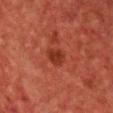workup: total-body-photography surveillance lesion; no biopsy
automated lesion analysis: a lesion area of about 4 mm², an eccentricity of roughly 0.6, and a shape-asymmetry score of about 0.2 (0 = symmetric); a nevus-likeness score of about 5/100 and a detector confidence of about 100 out of 100 that the crop contains a lesion
subject: male, approximately 50 years of age
lesion diameter: ~2.5 mm (longest diameter)
site: the chest
acquisition: ~15 mm tile from a whole-body skin photo